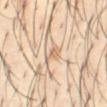No biopsy was performed on this lesion — it was imaged during a full skin examination and was not determined to be concerning.
On the abdomen.
A male patient aged 38–42.
A roughly 15 mm field-of-view crop from a total-body skin photograph.